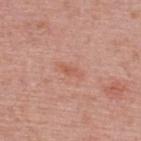| field | value |
|---|---|
| notes | catalogued during a skin exam; not biopsied |